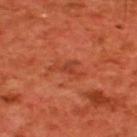The lesion was tiled from a total-body skin photograph and was not biopsied. A male patient, in their 60s. This is a cross-polarized tile. Measured at roughly 3.5 mm in maximum diameter. A 15 mm close-up tile from a total-body photography series done for melanoma screening. The lesion is located on the upper back.